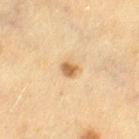{
  "biopsy_status": "not biopsied; imaged during a skin examination"
}